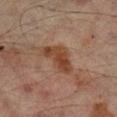Imaged during a routine full-body skin examination; the lesion was not biopsied and no histopathology is available.
On the leg.
The total-body-photography lesion software estimated a symmetry-axis asymmetry near 0.45. It also reported an automated nevus-likeness rating near 50 out of 100 and a detector confidence of about 100 out of 100 that the crop contains a lesion.
Imaged with cross-polarized lighting.
This image is a 15 mm lesion crop taken from a total-body photograph.
A male patient, roughly 65 years of age.